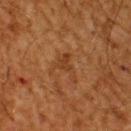Impression: Captured during whole-body skin photography for melanoma surveillance; the lesion was not biopsied. Acquisition and patient details: The recorded lesion diameter is about 3.5 mm. A male subject approximately 60 years of age. Automated image analysis of the tile measured a lesion color around L≈33 a*≈20 b*≈31 in CIELAB and about 6 CIELAB-L* units darker than the surrounding skin. The analysis additionally found an automated nevus-likeness rating near 0 out of 100 and lesion-presence confidence of about 100/100. Imaged with cross-polarized lighting. From the upper back. A lesion tile, about 15 mm wide, cut from a 3D total-body photograph.